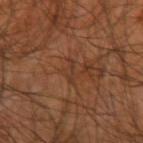Imaged during a routine full-body skin examination; the lesion was not biopsied and no histopathology is available.
About 2.5 mm across.
Located on the arm.
The subject is a male in their mid-40s.
A lesion tile, about 15 mm wide, cut from a 3D total-body photograph.